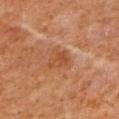follow-up: imaged on a skin check; not biopsied
patient: female, about 40 years old
site: the arm
lighting: cross-polarized
automated metrics: an automated nevus-likeness rating near 10 out of 100 and a detector confidence of about 100 out of 100 that the crop contains a lesion
lesion diameter: ≈3 mm
acquisition: 15 mm crop, total-body photography